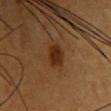Clinical impression: Captured during whole-body skin photography for melanoma surveillance; the lesion was not biopsied. Background: A lesion tile, about 15 mm wide, cut from a 3D total-body photograph. On the upper back. A female patient, about 45 years old.